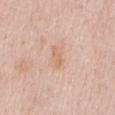{
  "biopsy_status": "not biopsied; imaged during a skin examination",
  "lesion_size": {
    "long_diameter_mm_approx": 2.5
  },
  "image": {
    "source": "total-body photography crop",
    "field_of_view_mm": 15
  },
  "lighting": "white-light",
  "patient": {
    "sex": "female",
    "age_approx": 50
  },
  "site": "mid back"
}